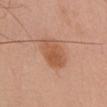Q: What are the patient's age and sex?
A: male, roughly 60 years of age
Q: Lesion size?
A: ≈4 mm
Q: What is the imaging modality?
A: ~15 mm crop, total-body skin-cancer survey
Q: Lesion location?
A: the chest